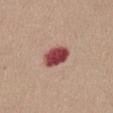- follow-up: total-body-photography surveillance lesion; no biopsy
- patient: female, aged around 45
- acquisition: ~15 mm crop, total-body skin-cancer survey
- site: the abdomen
- TBP lesion metrics: a classifier nevus-likeness of about 0/100 and a detector confidence of about 100 out of 100 that the crop contains a lesion